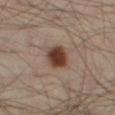workup = no biopsy performed (imaged during a skin exam); acquisition = 15 mm crop, total-body photography; TBP lesion metrics = an automated nevus-likeness rating near 100 out of 100; subject = male, approximately 40 years of age; site = the left thigh; lighting = cross-polarized.